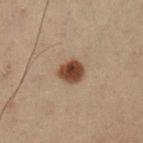Image and clinical context: Located on the left lower leg. The patient is a male in their mid-50s. Approximately 3 mm at its widest. The tile uses cross-polarized illumination. The lesion-visualizer software estimated a shape eccentricity near 0.5 and a shape-asymmetry score of about 0.2 (0 = symmetric). The analysis additionally found a classifier nevus-likeness of about 100/100 and a lesion-detection confidence of about 100/100. A roughly 15 mm field-of-view crop from a total-body skin photograph.